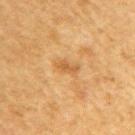{"biopsy_status": "not biopsied; imaged during a skin examination", "lesion_size": {"long_diameter_mm_approx": 3.0}, "patient": {"sex": "male", "age_approx": 50}, "site": "right upper arm", "automated_metrics": {"cielab_L": 47, "cielab_a": 18, "cielab_b": 37, "vs_skin_darker_L": 7.0, "vs_skin_contrast_norm": 6.0}, "image": {"source": "total-body photography crop", "field_of_view_mm": 15}}